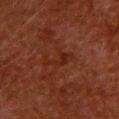  biopsy_status: not biopsied; imaged during a skin examination
  lesion_size:
    long_diameter_mm_approx: 4.0
  automated_metrics:
    shape_asymmetry: 0.45
    cielab_L: 21
    cielab_a: 22
    cielab_b: 25
    vs_skin_contrast_norm: 5.5
    nevus_likeness_0_100: 5
    lesion_detection_confidence_0_100: 100
  image:
    source: total-body photography crop
    field_of_view_mm: 15
  patient:
    sex: female
    age_approx: 50
  lighting: cross-polarized
  site: back